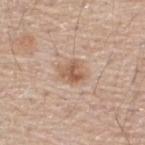Assessment: The lesion was photographed on a routine skin check and not biopsied; there is no pathology result. Context: A 15 mm close-up tile from a total-body photography series done for melanoma screening. Located on the upper back. Approximately 3 mm at its widest. Imaged with white-light lighting. A male subject, roughly 55 years of age. Automated tile analysis of the lesion measured an area of roughly 5.5 mm², an outline eccentricity of about 0.55 (0 = round, 1 = elongated), and a shape-asymmetry score of about 0.25 (0 = symmetric). It also reported a lesion color around L≈58 a*≈19 b*≈31 in CIELAB and about 10 CIELAB-L* units darker than the surrounding skin. It also reported border irregularity of about 3 on a 0–10 scale and radial color variation of about 1.5. And it measured a lesion-detection confidence of about 100/100.